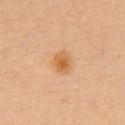Assessment:
Recorded during total-body skin imaging; not selected for excision or biopsy.
Acquisition and patient details:
A 15 mm crop from a total-body photograph taken for skin-cancer surveillance. A female patient aged 38–42. On the chest.